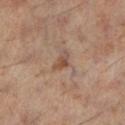follow-up = catalogued during a skin exam; not biopsied
image-analysis metrics = lesion-presence confidence of about 100/100
patient = male, roughly 60 years of age
location = the left lower leg
acquisition = ~15 mm tile from a whole-body skin photo
illumination = cross-polarized
diameter = about 2.5 mm